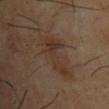Q: Is there a histopathology result?
A: imaged on a skin check; not biopsied
Q: What is the lesion's diameter?
A: ~6 mm (longest diameter)
Q: Patient demographics?
A: male, in their mid- to late 60s
Q: What kind of image is this?
A: ~15 mm crop, total-body skin-cancer survey
Q: Illumination type?
A: cross-polarized
Q: Where on the body is the lesion?
A: the chest